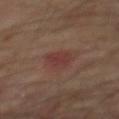biopsy status = imaged on a skin check; not biopsied | subject = male, aged approximately 65 | body site = the mid back | tile lighting = cross-polarized | image = total-body-photography crop, ~15 mm field of view | lesion diameter = about 3 mm.